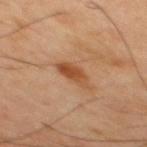| field | value |
|---|---|
| biopsy status | total-body-photography surveillance lesion; no biopsy |
| tile lighting | cross-polarized |
| lesion size | ≈3.5 mm |
| subject | male, aged approximately 45 |
| image | ~15 mm tile from a whole-body skin photo |
| body site | the mid back |